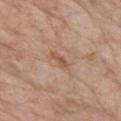Part of a total-body skin-imaging series; this lesion was reviewed on a skin check and was not flagged for biopsy. The patient is a female approximately 70 years of age. The tile uses white-light illumination. On the front of the torso. The recorded lesion diameter is about 3 mm. Cropped from a total-body skin-imaging series; the visible field is about 15 mm.